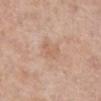Captured during whole-body skin photography for melanoma surveillance; the lesion was not biopsied. A region of skin cropped from a whole-body photographic capture, roughly 15 mm wide. A female patient, aged 63 to 67. The lesion is on the right lower leg. This is a white-light tile. The total-body-photography lesion software estimated a border-irregularity rating of about 2/10, a color-variation rating of about 1.5/10, and a peripheral color-asymmetry measure near 0.5. And it measured lesion-presence confidence of about 100/100.